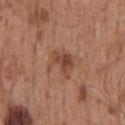This image is a 15 mm lesion crop taken from a total-body photograph.
The lesion's longest dimension is about 3.5 mm.
Automated image analysis of the tile measured a lesion color around L≈46 a*≈22 b*≈29 in CIELAB, a lesion–skin lightness drop of about 9, and a normalized lesion–skin contrast near 7. The analysis additionally found internal color variation of about 4 on a 0–10 scale and a peripheral color-asymmetry measure near 1.5. It also reported an automated nevus-likeness rating near 0 out of 100.
A male subject in their 60s.
On the mid back.
Imaged with white-light lighting.